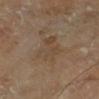No biopsy was performed on this lesion — it was imaged during a full skin examination and was not determined to be concerning.
Cropped from a total-body skin-imaging series; the visible field is about 15 mm.
The total-body-photography lesion software estimated a footprint of about 9.5 mm², an eccentricity of roughly 0.8, and two-axis asymmetry of about 0.25. The analysis additionally found a border-irregularity index near 4/10 and a within-lesion color-variation index near 3.5/10.
A male subject aged 63 to 67.
Approximately 5 mm at its widest.
Located on the right lower leg.